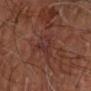  biopsy_status: not biopsied; imaged during a skin examination
  lesion_size:
    long_diameter_mm_approx: 3.5
  automated_metrics:
    cielab_L: 30
    cielab_a: 21
    cielab_b: 20
    vs_skin_darker_L: 5.0
    vs_skin_contrast_norm: 5.5
  image:
    source: total-body photography crop
    field_of_view_mm: 15
  patient:
    sex: male
    age_approx: 70
  site: right forearm